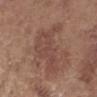The lesion was tiled from a total-body skin photograph and was not biopsied.
The lesion is on the right forearm.
Cropped from a total-body skin-imaging series; the visible field is about 15 mm.
An algorithmic analysis of the crop reported a footprint of about 18 mm², a shape eccentricity near 0.65, and a symmetry-axis asymmetry near 0.5. And it measured an automated nevus-likeness rating near 0 out of 100 and a lesion-detection confidence of about 100/100.
A female subject approximately 65 years of age.